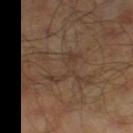Assessment: This lesion was catalogued during total-body skin photography and was not selected for biopsy. Acquisition and patient details: An algorithmic analysis of the crop reported a border-irregularity rating of about 9.5/10 and a color-variation rating of about 2/10. And it measured a classifier nevus-likeness of about 0/100 and a detector confidence of about 85 out of 100 that the crop contains a lesion. A male patient in their mid-40s. Cropped from a whole-body photographic skin survey; the tile spans about 15 mm. This is a cross-polarized tile. Located on the leg.